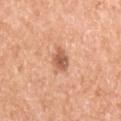notes: total-body-photography surveillance lesion; no biopsy
site: the left upper arm
patient: female, roughly 70 years of age
acquisition: 15 mm crop, total-body photography